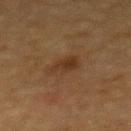| field | value |
|---|---|
| workup | imaged on a skin check; not biopsied |
| lesion diameter | ~3 mm (longest diameter) |
| site | the upper back |
| image source | total-body-photography crop, ~15 mm field of view |
| patient | female, aged 53–57 |
| lighting | cross-polarized illumination |